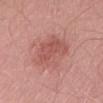Q: Was a biopsy performed?
A: no biopsy performed (imaged during a skin exam)
Q: Who is the patient?
A: male, aged approximately 55
Q: Lesion size?
A: about 6.5 mm
Q: What did automated image analysis measure?
A: an area of roughly 17 mm², an outline eccentricity of about 0.9 (0 = round, 1 = elongated), and a shape-asymmetry score of about 0.2 (0 = symmetric); a normalized border contrast of about 6; internal color variation of about 3.5 on a 0–10 scale and a peripheral color-asymmetry measure near 1
Q: How was this image acquired?
A: ~15 mm tile from a whole-body skin photo
Q: What lighting was used for the tile?
A: white-light illumination
Q: What is the anatomic site?
A: the lower back